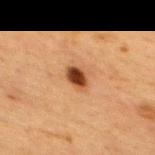| key | value |
|---|---|
| biopsy status | imaged on a skin check; not biopsied |
| illumination | cross-polarized |
| subject | female, in their mid- to late 50s |
| site | the upper back |
| image | ~15 mm crop, total-body skin-cancer survey |